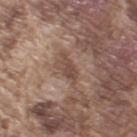Assessment:
The lesion was tiled from a total-body skin photograph and was not biopsied.
Background:
Captured under white-light illumination. The lesion is located on the arm. Longest diameter approximately 4 mm. The patient is a male in their mid-70s. A lesion tile, about 15 mm wide, cut from a 3D total-body photograph. The total-body-photography lesion software estimated a shape-asymmetry score of about 0.35 (0 = symmetric).